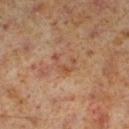The recorded lesion diameter is about 3 mm.
The patient is a male roughly 60 years of age.
The lesion is located on the left lower leg.
A roughly 15 mm field-of-view crop from a total-body skin photograph.